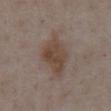{"biopsy_status": "not biopsied; imaged during a skin examination", "site": "abdomen", "image": {"source": "total-body photography crop", "field_of_view_mm": 15}, "patient": {"sex": "male", "age_approx": 75}}